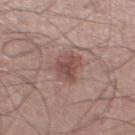size: ~3.5 mm (longest diameter) | subject: male, approximately 50 years of age | site: the leg | imaging modality: total-body-photography crop, ~15 mm field of view.